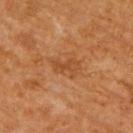Recorded during total-body skin imaging; not selected for excision or biopsy.
A male subject roughly 60 years of age.
The tile uses cross-polarized illumination.
An algorithmic analysis of the crop reported a lesion area of about 5 mm² and a shape eccentricity near 0.8. And it measured a lesion-to-skin contrast of about 5.5 (normalized; higher = more distinct). The analysis additionally found border irregularity of about 4.5 on a 0–10 scale, a within-lesion color-variation index near 2/10, and radial color variation of about 0.5.
The recorded lesion diameter is about 3.5 mm.
This image is a 15 mm lesion crop taken from a total-body photograph.
From the upper back.